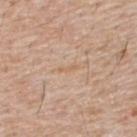subject=male, aged 53–57; acquisition=~15 mm tile from a whole-body skin photo; location=the upper back.